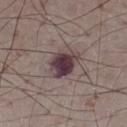Q: Was a biopsy performed?
A: total-body-photography surveillance lesion; no biopsy
Q: Who is the patient?
A: male, in their mid- to late 70s
Q: Lesion location?
A: the leg
Q: Lesion size?
A: ~4 mm (longest diameter)
Q: What is the imaging modality?
A: 15 mm crop, total-body photography
Q: Automated lesion metrics?
A: a lesion area of about 9.5 mm² and two-axis asymmetry of about 0.2; a lesion color around L≈37 a*≈17 b*≈11 in CIELAB, roughly 15 lightness units darker than nearby skin, and a normalized lesion–skin contrast near 13; border irregularity of about 2.5 on a 0–10 scale and a peripheral color-asymmetry measure near 2.5; a nevus-likeness score of about 5/100 and a lesion-detection confidence of about 100/100
Q: Illumination type?
A: white-light illumination